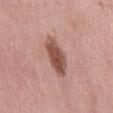No biopsy was performed on this lesion — it was imaged during a full skin examination and was not determined to be concerning. Cropped from a whole-body photographic skin survey; the tile spans about 15 mm. The lesion is on the mid back. A male subject roughly 70 years of age. Measured at roughly 5 mm in maximum diameter.